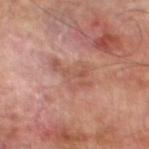| key | value |
|---|---|
| biopsy status | imaged on a skin check; not biopsied |
| tile lighting | cross-polarized illumination |
| subject | male, aged around 70 |
| lesion size | ≈5 mm |
| anatomic site | the left lower leg |
| acquisition | 15 mm crop, total-body photography |